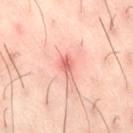biopsy status=total-body-photography surveillance lesion; no biopsy